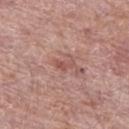{
  "biopsy_status": "not biopsied; imaged during a skin examination",
  "image": {
    "source": "total-body photography crop",
    "field_of_view_mm": 15
  },
  "patient": {
    "sex": "male",
    "age_approx": 65
  },
  "lighting": "white-light",
  "lesion_size": {
    "long_diameter_mm_approx": 2.5
  },
  "site": "left thigh"
}